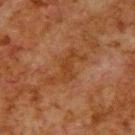follow-up — no biopsy performed (imaged during a skin exam); image source — 15 mm crop, total-body photography; patient — male, in their 80s; automated metrics — an area of roughly 9 mm² and a symmetry-axis asymmetry near 0.6; lesion diameter — ≈5.5 mm; lighting — cross-polarized illumination; site — the upper back.